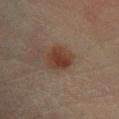Captured during whole-body skin photography for melanoma surveillance; the lesion was not biopsied. The patient is a female in their 80s. Cropped from a total-body skin-imaging series; the visible field is about 15 mm. Located on the leg.